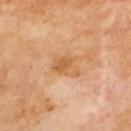The lesion was photographed on a routine skin check and not biopsied; there is no pathology result.
The lesion's longest dimension is about 4 mm.
Cropped from a whole-body photographic skin survey; the tile spans about 15 mm.
A male patient, in their 70s.
The tile uses cross-polarized illumination.
On the back.